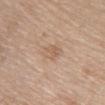Recorded during total-body skin imaging; not selected for excision or biopsy.
Measured at roughly 3 mm in maximum diameter.
A lesion tile, about 15 mm wide, cut from a 3D total-body photograph.
The lesion is located on the chest.
Automated image analysis of the tile measured an area of roughly 5 mm², a shape eccentricity near 0.75, and a symmetry-axis asymmetry near 0.3. And it measured border irregularity of about 3 on a 0–10 scale, a within-lesion color-variation index near 3/10, and radial color variation of about 1.
A female subject about 50 years old.